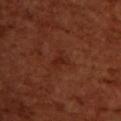{
  "biopsy_status": "not biopsied; imaged during a skin examination",
  "site": "upper back",
  "image": {
    "source": "total-body photography crop",
    "field_of_view_mm": 15
  },
  "patient": {
    "sex": "female",
    "age_approx": 55
  }
}